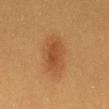notes: imaged on a skin check; not biopsied | TBP lesion metrics: an area of roughly 10 mm², an eccentricity of roughly 0.85, and a symmetry-axis asymmetry near 0.2; an average lesion color of about L≈39 a*≈19 b*≈33 (CIELAB), a lesion–skin lightness drop of about 7, and a lesion-to-skin contrast of about 6.5 (normalized; higher = more distinct); a border-irregularity index near 2.5/10, a within-lesion color-variation index near 2.5/10, and radial color variation of about 1; an automated nevus-likeness rating near 90 out of 100 and a lesion-detection confidence of about 100/100 | anatomic site: the mid back | illumination: cross-polarized | patient: female, about 40 years old | lesion diameter: ≈5 mm | imaging modality: total-body-photography crop, ~15 mm field of view.